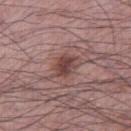Background: A male subject, roughly 50 years of age. Captured under white-light illumination. Longest diameter approximately 3.5 mm. On the right thigh. A roughly 15 mm field-of-view crop from a total-body skin photograph.